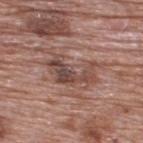Impression: Imaged during a routine full-body skin examination; the lesion was not biopsied and no histopathology is available. Acquisition and patient details: The patient is a male aged 68–72. A lesion tile, about 15 mm wide, cut from a 3D total-body photograph. Longest diameter approximately 6 mm. On the upper back. The tile uses white-light illumination.